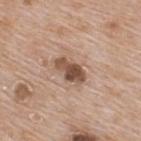{"biopsy_status": "not biopsied; imaged during a skin examination", "image": {"source": "total-body photography crop", "field_of_view_mm": 15}, "site": "upper back", "lighting": "white-light", "lesion_size": {"long_diameter_mm_approx": 4.0}, "patient": {"sex": "male", "age_approx": 65}}